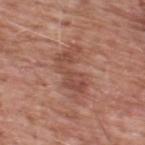Clinical impression:
Captured during whole-body skin photography for melanoma surveillance; the lesion was not biopsied.
Acquisition and patient details:
The subject is a male about 60 years old. Cropped from a total-body skin-imaging series; the visible field is about 15 mm. From the upper back. Imaged with white-light lighting. An algorithmic analysis of the crop reported a footprint of about 13 mm² and an eccentricity of roughly 0.9. It also reported an average lesion color of about L≈49 a*≈23 b*≈27 (CIELAB) and a normalized lesion–skin contrast near 6.5. The software also gave border irregularity of about 6.5 on a 0–10 scale, a color-variation rating of about 3/10, and radial color variation of about 1.